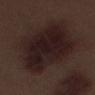Impression: The lesion was photographed on a routine skin check and not biopsied; there is no pathology result. Acquisition and patient details: Approximately 9 mm at its widest. Imaged with white-light lighting. A male subject aged 68 to 72. The lesion-visualizer software estimated a border-irregularity rating of about 2/10, a within-lesion color-variation index near 4/10, and radial color variation of about 1. And it measured a nevus-likeness score of about 0/100. Cropped from a whole-body photographic skin survey; the tile spans about 15 mm. On the right thigh.